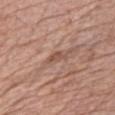Part of a total-body skin-imaging series; this lesion was reviewed on a skin check and was not flagged for biopsy.
Measured at roughly 2.5 mm in maximum diameter.
This image is a 15 mm lesion crop taken from a total-body photograph.
Captured under white-light illumination.
The lesion-visualizer software estimated a footprint of about 2.5 mm², a shape eccentricity near 0.85, and a shape-asymmetry score of about 0.45 (0 = symmetric). It also reported an average lesion color of about L≈52 a*≈21 b*≈28 (CIELAB), about 8 CIELAB-L* units darker than the surrounding skin, and a normalized border contrast of about 6. It also reported a nevus-likeness score of about 0/100 and lesion-presence confidence of about 55/100.
A female patient, approximately 65 years of age.
From the chest.